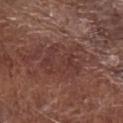The lesion was tiled from a total-body skin photograph and was not biopsied. The lesion is located on the right forearm. A roughly 15 mm field-of-view crop from a total-body skin photograph. Approximately 5 mm at its widest. The patient is a male in their 70s.